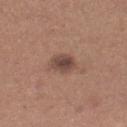follow-up: total-body-photography surveillance lesion; no biopsy
automated metrics: an average lesion color of about L≈45 a*≈19 b*≈24 (CIELAB), roughly 11 lightness units darker than nearby skin, and a lesion-to-skin contrast of about 9 (normalized; higher = more distinct); a classifier nevus-likeness of about 55/100 and a lesion-detection confidence of about 100/100
body site: the right lower leg
imaging modality: ~15 mm crop, total-body skin-cancer survey
illumination: white-light
patient: female, aged 28 to 32